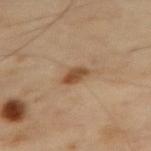| field | value |
|---|---|
| workup | imaged on a skin check; not biopsied |
| patient | male, aged 68 to 72 |
| location | the right thigh |
| diameter | ~3 mm (longest diameter) |
| image | ~15 mm tile from a whole-body skin photo |
| tile lighting | cross-polarized illumination |